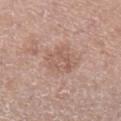Q: Was a biopsy performed?
A: catalogued during a skin exam; not biopsied
Q: What are the patient's age and sex?
A: female, roughly 50 years of age
Q: What lighting was used for the tile?
A: white-light illumination
Q: Lesion location?
A: the leg
Q: What is the imaging modality?
A: ~15 mm crop, total-body skin-cancer survey